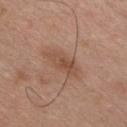workup: catalogued during a skin exam; not biopsied | acquisition: 15 mm crop, total-body photography | diameter: ~4.5 mm (longest diameter) | patient: male, aged around 45 | anatomic site: the chest.